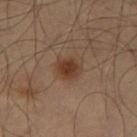– workup · imaged on a skin check; not biopsied
– image source · total-body-photography crop, ~15 mm field of view
– body site · the leg
– TBP lesion metrics · a lesion area of about 7 mm², an eccentricity of roughly 0.55, and two-axis asymmetry of about 0.15; a mean CIELAB color near L≈39 a*≈19 b*≈29, roughly 9 lightness units darker than nearby skin, and a normalized border contrast of about 8.5
– lighting · cross-polarized
– lesion diameter · ≈3 mm